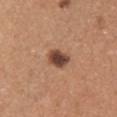  biopsy_status: not biopsied; imaged during a skin examination
  site: right upper arm
  lesion_size:
    long_diameter_mm_approx: 3.0
  image:
    source: total-body photography crop
    field_of_view_mm: 15
  lighting: white-light
  patient:
    sex: female
    age_approx: 55